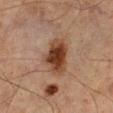Imaged during a routine full-body skin examination; the lesion was not biopsied and no histopathology is available.
The patient is a male aged 63–67.
The lesion's longest dimension is about 4.5 mm.
The tile uses cross-polarized illumination.
On the leg.
This image is a 15 mm lesion crop taken from a total-body photograph.